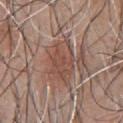Assessment: No biopsy was performed on this lesion — it was imaged during a full skin examination and was not determined to be concerning. Clinical summary: The tile uses white-light illumination. The patient is a male aged 43 to 47. The lesion is on the chest. Approximately 6 mm at its widest. A close-up tile cropped from a whole-body skin photograph, about 15 mm across.